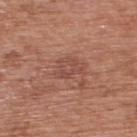The lesion was tiled from a total-body skin photograph and was not biopsied. The lesion's longest dimension is about 3.5 mm. A male subject, in their 70s. A 15 mm close-up tile from a total-body photography series done for melanoma screening. The lesion is on the upper back. Automated image analysis of the tile measured a footprint of about 6 mm², an outline eccentricity of about 0.7 (0 = round, 1 = elongated), and two-axis asymmetry of about 0.35. The software also gave a border-irregularity index near 4/10 and radial color variation of about 1. The software also gave a nevus-likeness score of about 0/100 and lesion-presence confidence of about 100/100. This is a white-light tile.